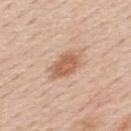Notes:
– notes: imaged on a skin check; not biopsied
– size: ~4 mm (longest diameter)
– subject: male, in their 60s
– automated metrics: a footprint of about 7.5 mm², an outline eccentricity of about 0.8 (0 = round, 1 = elongated), and a symmetry-axis asymmetry near 0.2; border irregularity of about 2.5 on a 0–10 scale
– anatomic site: the mid back
– acquisition: 15 mm crop, total-body photography
– illumination: white-light